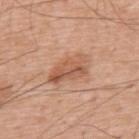Q: Who is the patient?
A: male, in their 60s
Q: What is the lesion's diameter?
A: ~5 mm (longest diameter)
Q: What lighting was used for the tile?
A: white-light illumination
Q: What kind of image is this?
A: ~15 mm crop, total-body skin-cancer survey
Q: What is the anatomic site?
A: the upper back